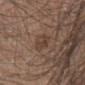notes: no biopsy performed (imaged during a skin exam) | lesion size: ~3.5 mm (longest diameter) | patient: male, roughly 45 years of age | anatomic site: the right lower leg | imaging modality: ~15 mm tile from a whole-body skin photo.